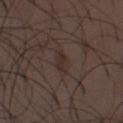The lesion was tiled from a total-body skin photograph and was not biopsied. A region of skin cropped from a whole-body photographic capture, roughly 15 mm wide. The recorded lesion diameter is about 2.5 mm. The lesion is on the front of the torso. The lesion-visualizer software estimated a mean CIELAB color near L≈28 a*≈14 b*≈18. And it measured a border-irregularity index near 3/10 and peripheral color asymmetry of about 0. A male subject, aged 28–32. This is a white-light tile.